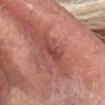{
  "automated_metrics": {
    "eccentricity": 0.9,
    "shape_asymmetry": 0.2,
    "cielab_L": 45,
    "cielab_a": 28,
    "cielab_b": 26,
    "vs_skin_darker_L": 9.0,
    "vs_skin_contrast_norm": 6.5,
    "nevus_likeness_0_100": 0,
    "lesion_detection_confidence_0_100": 90
  },
  "patient": {
    "sex": "female",
    "age_approx": 55
  },
  "lighting": "white-light",
  "image": {
    "source": "total-body photography crop",
    "field_of_view_mm": 15
  },
  "site": "head or neck",
  "lesion_size": {
    "long_diameter_mm_approx": 3.0
  }
}